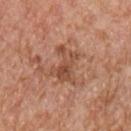| key | value |
|---|---|
| notes | no biopsy performed (imaged during a skin exam) |
| subject | male, aged 63–67 |
| acquisition | ~15 mm tile from a whole-body skin photo |
| location | the chest |